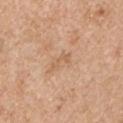patient: male, approximately 55 years of age
body site: the left upper arm
tile lighting: white-light illumination
image: 15 mm crop, total-body photography